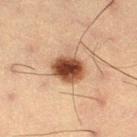notes=imaged on a skin check; not biopsied | TBP lesion metrics=an area of roughly 10 mm², a shape eccentricity near 0.6, and a symmetry-axis asymmetry near 0.1; lesion-presence confidence of about 100/100 | lesion size=~4 mm (longest diameter) | image=15 mm crop, total-body photography | patient=male, in their mid-50s | anatomic site=the left thigh.